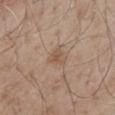acquisition = ~15 mm crop, total-body skin-cancer survey
lesion size = ~2.5 mm (longest diameter)
anatomic site = the mid back
subject = male, approximately 55 years of age
illumination = white-light illumination